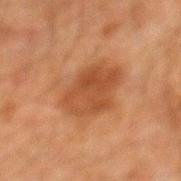Imaged during a routine full-body skin examination; the lesion was not biopsied and no histopathology is available. About 6.5 mm across. Captured under cross-polarized illumination. The lesion-visualizer software estimated a footprint of about 24 mm² and a shape eccentricity near 0.65. The software also gave a lesion color around L≈39 a*≈20 b*≈31 in CIELAB, roughly 7 lightness units darker than nearby skin, and a normalized border contrast of about 6.5. The analysis additionally found border irregularity of about 3.5 on a 0–10 scale and internal color variation of about 3.5 on a 0–10 scale. The software also gave a nevus-likeness score of about 80/100 and a detector confidence of about 100 out of 100 that the crop contains a lesion. The lesion is located on the mid back. A 15 mm close-up extracted from a 3D total-body photography capture. The patient is a male aged 58–62.